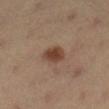Background:
A close-up tile cropped from a whole-body skin photograph, about 15 mm across. Approximately 3 mm at its widest. Located on the right lower leg. An algorithmic analysis of the crop reported a lesion–skin lightness drop of about 11 and a lesion-to-skin contrast of about 9.5 (normalized; higher = more distinct). And it measured internal color variation of about 2 on a 0–10 scale and a peripheral color-asymmetry measure near 0.5. A female patient aged around 35.